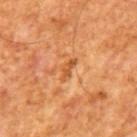| feature | finding |
|---|---|
| workup | catalogued during a skin exam; not biopsied |
| patient | male, approximately 65 years of age |
| image | ~15 mm crop, total-body skin-cancer survey |
| lesion size | ~3 mm (longest diameter) |
| tile lighting | cross-polarized illumination |